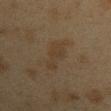About 4 mm across.
The subject is a female about 40 years old.
Imaged with cross-polarized lighting.
Located on the left upper arm.
A region of skin cropped from a whole-body photographic capture, roughly 15 mm wide.